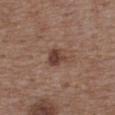The lesion was photographed on a routine skin check and not biopsied; there is no pathology result. The lesion-visualizer software estimated an area of roughly 6 mm², a shape eccentricity near 0.8, and a shape-asymmetry score of about 0.25 (0 = symmetric). The software also gave a lesion color around L≈42 a*≈19 b*≈24 in CIELAB, roughly 10 lightness units darker than nearby skin, and a normalized lesion–skin contrast near 8.5. The analysis additionally found border irregularity of about 2.5 on a 0–10 scale and radial color variation of about 1.5. It also reported a classifier nevus-likeness of about 85/100 and a lesion-detection confidence of about 100/100. A 15 mm crop from a total-body photograph taken for skin-cancer surveillance. On the upper back. A male patient approximately 50 years of age.